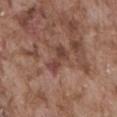Findings:
• workup · imaged on a skin check; not biopsied
• location · the front of the torso
• diameter · ≈3.5 mm
• patient · male, aged 73 to 77
• acquisition · ~15 mm crop, total-body skin-cancer survey